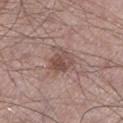| feature | finding |
|---|---|
| size | ≈3 mm |
| patient | male, aged around 65 |
| image source | ~15 mm crop, total-body skin-cancer survey |
| illumination | white-light illumination |
| body site | the left lower leg |